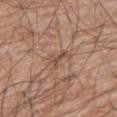* biopsy status: imaged on a skin check; not biopsied